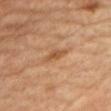follow-up: total-body-photography surveillance lesion; no biopsy
image-analysis metrics: an outline eccentricity of about 0.85 (0 = round, 1 = elongated) and a symmetry-axis asymmetry near 0.3; a nevus-likeness score of about 5/100 and a lesion-detection confidence of about 100/100
lesion size: about 3.5 mm
site: the chest
image: 15 mm crop, total-body photography
subject: female, in their 60s